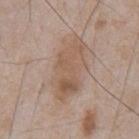<lesion>
<biopsy_status>not biopsied; imaged during a skin examination</biopsy_status>
<lesion_size>
  <long_diameter_mm_approx>7.5</long_diameter_mm_approx>
</lesion_size>
<lighting>white-light</lighting>
<patient>
  <sex>male</sex>
  <age_approx>65</age_approx>
</patient>
<image>
  <source>total-body photography crop</source>
  <field_of_view_mm>15</field_of_view_mm>
</image>
<site>chest</site>
</lesion>